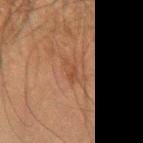Clinical impression: Recorded during total-body skin imaging; not selected for excision or biopsy. Image and clinical context: An algorithmic analysis of the crop reported about 5 CIELAB-L* units darker than the surrounding skin and a lesion-to-skin contrast of about 5 (normalized; higher = more distinct). The analysis additionally found a border-irregularity index near 4/10 and a peripheral color-asymmetry measure near 1. It also reported a nevus-likeness score of about 5/100. From the left forearm. This image is a 15 mm lesion crop taken from a total-body photograph. The recorded lesion diameter is about 2.5 mm. This is a cross-polarized tile. A male patient, roughly 60 years of age.